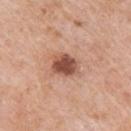{"biopsy_status": "not biopsied; imaged during a skin examination", "patient": {"sex": "male", "age_approx": 65}, "image": {"source": "total-body photography crop", "field_of_view_mm": 15}, "site": "arm"}